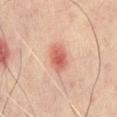| key | value |
|---|---|
| workup | imaged on a skin check; not biopsied |
| location | the chest |
| subject | male, about 60 years old |
| image | ~15 mm tile from a whole-body skin photo |